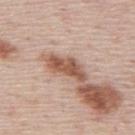  biopsy_status: not biopsied; imaged during a skin examination
  site: mid back
  patient:
    sex: male
    age_approx: 45
  automated_metrics:
    area_mm2_approx: 7.0
    eccentricity: 0.9
    shape_asymmetry: 0.3
    vs_skin_darker_L: 15.0
    vs_skin_contrast_norm: 10.0
    border_irregularity_0_10: 3.5
    color_variation_0_10: 3.0
    peripheral_color_asymmetry: 1.0
  image:
    source: total-body photography crop
    field_of_view_mm: 15